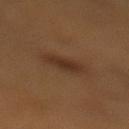Impression: Recorded during total-body skin imaging; not selected for excision or biopsy. Image and clinical context: Cropped from a total-body skin-imaging series; the visible field is about 15 mm. Measured at roughly 3.5 mm in maximum diameter. An algorithmic analysis of the crop reported an average lesion color of about L≈31 a*≈17 b*≈27 (CIELAB) and a lesion-to-skin contrast of about 7 (normalized; higher = more distinct). The analysis additionally found a detector confidence of about 100 out of 100 that the crop contains a lesion. The lesion is located on the left lower leg. A male patient, approximately 55 years of age. Imaged with cross-polarized lighting.